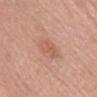Imaged during a routine full-body skin examination; the lesion was not biopsied and no histopathology is available. Cropped from a whole-body photographic skin survey; the tile spans about 15 mm. The subject is a female roughly 65 years of age. On the head or neck. The lesion's longest dimension is about 3.5 mm. Captured under white-light illumination.